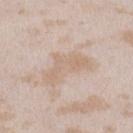Recorded during total-body skin imaging; not selected for excision or biopsy. Cropped from a total-body skin-imaging series; the visible field is about 15 mm. Imaged with white-light lighting. The lesion's longest dimension is about 5.5 mm. On the left lower leg. Automated image analysis of the tile measured a lesion color around L≈66 a*≈14 b*≈28 in CIELAB, a lesion–skin lightness drop of about 7, and a normalized lesion–skin contrast near 5. It also reported a nevus-likeness score of about 0/100 and a lesion-detection confidence of about 90/100. A female subject, aged 23 to 27.